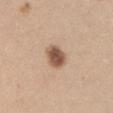Q: Is there a histopathology result?
A: no biopsy performed (imaged during a skin exam)
Q: What lighting was used for the tile?
A: white-light illumination
Q: How was this image acquired?
A: total-body-photography crop, ~15 mm field of view
Q: Patient demographics?
A: female, approximately 25 years of age
Q: Lesion location?
A: the arm
Q: Automated lesion metrics?
A: about 16 CIELAB-L* units darker than the surrounding skin and a normalized lesion–skin contrast near 10.5; a nevus-likeness score of about 100/100 and lesion-presence confidence of about 100/100